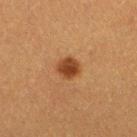site: right thigh
automated_metrics:
  area_mm2_approx: 5.5
  cielab_L: 35
  cielab_a: 21
  cielab_b: 32
lighting: cross-polarized
patient:
  sex: female
  age_approx: 40
image:
  source: total-body photography crop
  field_of_view_mm: 15
lesion_size:
  long_diameter_mm_approx: 2.5